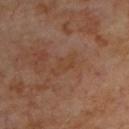Impression:
No biopsy was performed on this lesion — it was imaged during a full skin examination and was not determined to be concerning.
Context:
A 15 mm close-up tile from a total-body photography series done for melanoma screening. A male subject, approximately 70 years of age. The recorded lesion diameter is about 3.5 mm. Located on the upper back. Captured under cross-polarized illumination.